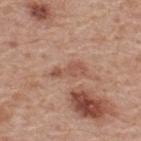– biopsy status · no biopsy performed (imaged during a skin exam)
– tile lighting · white-light illumination
– subject · male, aged approximately 60
– image · total-body-photography crop, ~15 mm field of view
– site · the upper back